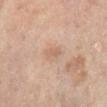{
  "biopsy_status": "not biopsied; imaged during a skin examination"
}